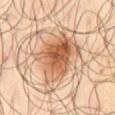No biopsy was performed on this lesion — it was imaged during a full skin examination and was not determined to be concerning.
Cropped from a total-body skin-imaging series; the visible field is about 15 mm.
A male patient approximately 65 years of age.
Located on the abdomen.
The lesion-visualizer software estimated a lesion color around L≈56 a*≈21 b*≈34 in CIELAB, about 14 CIELAB-L* units darker than the surrounding skin, and a normalized border contrast of about 9.